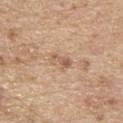follow-up=no biopsy performed (imaged during a skin exam).